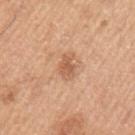{"biopsy_status": "not biopsied; imaged during a skin examination", "automated_metrics": {"eccentricity": 0.8, "shape_asymmetry": 0.35, "border_irregularity_0_10": 4.0, "peripheral_color_asymmetry": 0.5, "nevus_likeness_0_100": 60, "lesion_detection_confidence_0_100": 100}, "site": "arm", "lighting": "white-light", "lesion_size": {"long_diameter_mm_approx": 3.0}, "image": {"source": "total-body photography crop", "field_of_view_mm": 15}, "patient": {"sex": "male", "age_approx": 50}}